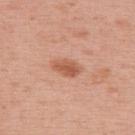Impression: Part of a total-body skin-imaging series; this lesion was reviewed on a skin check and was not flagged for biopsy. Background: The lesion's longest dimension is about 3.5 mm. A female patient, about 50 years old. A lesion tile, about 15 mm wide, cut from a 3D total-body photograph. Automated tile analysis of the lesion measured an area of roughly 5.5 mm² and two-axis asymmetry of about 0.2. The analysis additionally found a border-irregularity rating of about 2/10 and a within-lesion color-variation index near 2.5/10. And it measured a lesion-detection confidence of about 100/100. The lesion is located on the upper back.